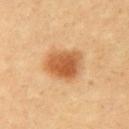biopsy status=imaged on a skin check; not biopsied
illumination=cross-polarized illumination
patient=female, approximately 35 years of age
location=the left upper arm
TBP lesion metrics=an area of roughly 13 mm², an eccentricity of roughly 0.45, and two-axis asymmetry of about 0.25; a lesion color around L≈57 a*≈25 b*≈41 in CIELAB and about 14 CIELAB-L* units darker than the surrounding skin
acquisition=~15 mm crop, total-body skin-cancer survey